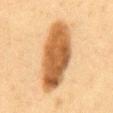| key | value |
|---|---|
| notes | imaged on a skin check; not biopsied |
| acquisition | ~15 mm crop, total-body skin-cancer survey |
| subject | female, in their 50s |
| tile lighting | cross-polarized |
| body site | the abdomen |